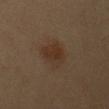<case>
<biopsy_status>not biopsied; imaged during a skin examination</biopsy_status>
<patient>
  <sex>male</sex>
  <age_approx>45</age_approx>
</patient>
<image>
  <source>total-body photography crop</source>
  <field_of_view_mm>15</field_of_view_mm>
</image>
<lesion_size>
  <long_diameter_mm_approx>4.0</long_diameter_mm_approx>
</lesion_size>
<site>left upper arm</site>
<lighting>cross-polarized</lighting>
</case>